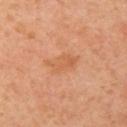| field | value |
|---|---|
| subject | female, roughly 40 years of age |
| site | the left upper arm |
| acquisition | total-body-photography crop, ~15 mm field of view |